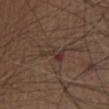Assessment: The lesion was tiled from a total-body skin photograph and was not biopsied. Background: Measured at roughly 3.5 mm in maximum diameter. A 15 mm close-up tile from a total-body photography series done for melanoma screening. The lesion is located on the chest. A male subject aged 73–77.